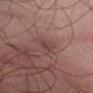Part of a total-body skin-imaging series; this lesion was reviewed on a skin check and was not flagged for biopsy. The subject is a male aged 48–52. The lesion's longest dimension is about 2.5 mm. The lesion is on the left thigh. Captured under white-light illumination. A close-up tile cropped from a whole-body skin photograph, about 15 mm across. An algorithmic analysis of the crop reported a shape eccentricity near 0.9 and two-axis asymmetry of about 0.4. The analysis additionally found a border-irregularity index near 4/10, a color-variation rating of about 0.5/10, and radial color variation of about 0.